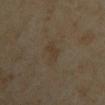{"biopsy_status": "not biopsied; imaged during a skin examination"}